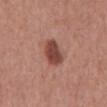Impression:
No biopsy was performed on this lesion — it was imaged during a full skin examination and was not determined to be concerning.
Context:
About 3.5 mm across. A lesion tile, about 15 mm wide, cut from a 3D total-body photograph. A male subject roughly 70 years of age. On the back. An algorithmic analysis of the crop reported a lesion color around L≈44 a*≈25 b*≈26 in CIELAB, about 14 CIELAB-L* units darker than the surrounding skin, and a lesion-to-skin contrast of about 10 (normalized; higher = more distinct). This is a white-light tile.